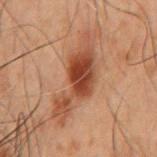Automated image analysis of the tile measured a mean CIELAB color near L≈36 a*≈20 b*≈27, about 10 CIELAB-L* units darker than the surrounding skin, and a normalized lesion–skin contrast near 9.
Cropped from a whole-body photographic skin survey; the tile spans about 15 mm.
A male patient aged 48–52.
Longest diameter approximately 8.5 mm.
The tile uses cross-polarized illumination.
The lesion is located on the mid back.